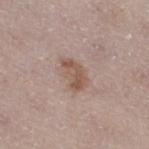Imaged during a routine full-body skin examination; the lesion was not biopsied and no histopathology is available. The subject is a female in their 50s. A close-up tile cropped from a whole-body skin photograph, about 15 mm across. The recorded lesion diameter is about 4 mm. On the right thigh.